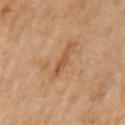Captured during whole-body skin photography for melanoma surveillance; the lesion was not biopsied. The subject is a female roughly 70 years of age. Imaged with cross-polarized lighting. Cropped from a total-body skin-imaging series; the visible field is about 15 mm. On the left upper arm. Automated tile analysis of the lesion measured about 8 CIELAB-L* units darker than the surrounding skin and a lesion-to-skin contrast of about 6 (normalized; higher = more distinct).